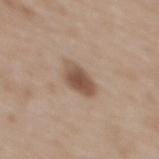notes: imaged on a skin check; not biopsied | automated metrics: a shape eccentricity near 0.8 and two-axis asymmetry of about 0.2; a lesion-detection confidence of about 100/100 | diameter: about 4 mm | patient: female, roughly 50 years of age | image: ~15 mm crop, total-body skin-cancer survey | site: the back | tile lighting: white-light illumination.